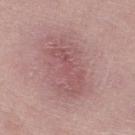- notes · catalogued during a skin exam; not biopsied
- anatomic site · the right lower leg
- subject · female, in their 50s
- tile lighting · white-light
- diameter · ≈6.5 mm
- acquisition · total-body-photography crop, ~15 mm field of view
- automated lesion analysis · a lesion area of about 17 mm², a shape eccentricity near 0.9, and a symmetry-axis asymmetry near 0.3; a lesion color around L≈54 a*≈23 b*≈19 in CIELAB and roughly 8 lightness units darker than nearby skin; a within-lesion color-variation index near 3.5/10; a nevus-likeness score of about 0/100 and a lesion-detection confidence of about 100/100